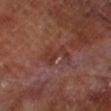The lesion was photographed on a routine skin check and not biopsied; there is no pathology result.
On the right lower leg.
The recorded lesion diameter is about 4 mm.
A region of skin cropped from a whole-body photographic capture, roughly 15 mm wide.
A male subject aged 68–72.
Imaged with cross-polarized lighting.